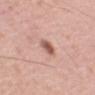{"image": {"source": "total-body photography crop", "field_of_view_mm": 15}, "lighting": "white-light", "site": "left upper arm", "patient": {"sex": "male", "age_approx": 55}, "lesion_size": {"long_diameter_mm_approx": 2.5}}